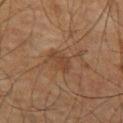workup: no biopsy performed (imaged during a skin exam) | location: the leg | lighting: cross-polarized illumination | patient: male, roughly 60 years of age | diameter: about 3 mm | automated lesion analysis: a mean CIELAB color near L≈37 a*≈18 b*≈28 and a normalized border contrast of about 6; border irregularity of about 5 on a 0–10 scale and a color-variation rating of about 1/10; a classifier nevus-likeness of about 0/100 and a lesion-detection confidence of about 100/100 | image source: ~15 mm crop, total-body skin-cancer survey.